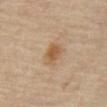An algorithmic analysis of the crop reported an area of roughly 5 mm², a shape eccentricity near 0.75, and two-axis asymmetry of about 0.25. The software also gave a lesion color around L≈52 a*≈17 b*≈33 in CIELAB and about 9 CIELAB-L* units darker than the surrounding skin. And it measured an automated nevus-likeness rating near 90 out of 100 and lesion-presence confidence of about 100/100. A region of skin cropped from a whole-body photographic capture, roughly 15 mm wide. The recorded lesion diameter is about 3 mm. On the abdomen. A male subject, in their mid- to late 60s.